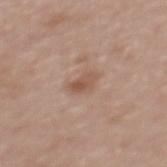Clinical summary: This is a white-light tile. The patient is a female aged 58–62. Automated image analysis of the tile measured a classifier nevus-likeness of about 35/100 and a detector confidence of about 100 out of 100 that the crop contains a lesion. A roughly 15 mm field-of-view crop from a total-body skin photograph. On the upper back.